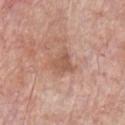Case summary:
– follow-up — total-body-photography surveillance lesion; no biopsy
– illumination — white-light illumination
– image source — ~15 mm crop, total-body skin-cancer survey
– patient — male, aged around 65
– body site — the front of the torso